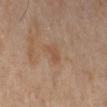Q: Is there a histopathology result?
A: imaged on a skin check; not biopsied
Q: Illumination type?
A: cross-polarized illumination
Q: Lesion location?
A: the right lower leg
Q: How was this image acquired?
A: ~15 mm tile from a whole-body skin photo
Q: Automated lesion metrics?
A: internal color variation of about 1.5 on a 0–10 scale and peripheral color asymmetry of about 0.5
Q: Who is the patient?
A: female, aged 48–52